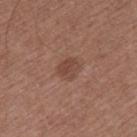Imaged during a routine full-body skin examination; the lesion was not biopsied and no histopathology is available. A male subject, aged 73 to 77. Located on the right thigh. A roughly 15 mm field-of-view crop from a total-body skin photograph.